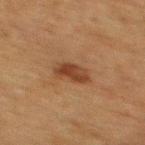The lesion was photographed on a routine skin check and not biopsied; there is no pathology result.
From the mid back.
About 3.5 mm across.
A 15 mm crop from a total-body photograph taken for skin-cancer surveillance.
An algorithmic analysis of the crop reported border irregularity of about 2.5 on a 0–10 scale, a within-lesion color-variation index near 3/10, and peripheral color asymmetry of about 1. The analysis additionally found a classifier nevus-likeness of about 95/100.
Captured under cross-polarized illumination.
A male patient, aged 48 to 52.